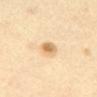| feature | finding |
|---|---|
| notes | total-body-photography surveillance lesion; no biopsy |
| lesion size | about 2.5 mm |
| subject | female, about 60 years old |
| site | the abdomen |
| lighting | cross-polarized |
| image source | total-body-photography crop, ~15 mm field of view |